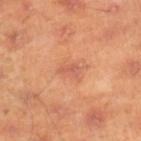Findings:
- imaging modality — 15 mm crop, total-body photography
- patient — male, approximately 45 years of age
- illumination — cross-polarized illumination
- body site — the right lower leg